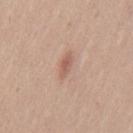Case summary:
• notes · catalogued during a skin exam; not biopsied
• image source · total-body-photography crop, ~15 mm field of view
• patient · male, in their mid-40s
• illumination · white-light illumination
• body site · the mid back